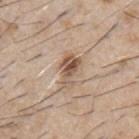Recorded during total-body skin imaging; not selected for excision or biopsy.
A 15 mm close-up tile from a total-body photography series done for melanoma screening.
A male subject, approximately 60 years of age.
The total-body-photography lesion software estimated a lesion area of about 6.5 mm², a shape eccentricity near 0.85, and two-axis asymmetry of about 0.35. And it measured a lesion–skin lightness drop of about 12.
Captured under white-light illumination.
On the chest.